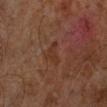Q: Was a biopsy performed?
A: imaged on a skin check; not biopsied
Q: How was this image acquired?
A: ~15 mm tile from a whole-body skin photo
Q: Where on the body is the lesion?
A: the left lower leg
Q: Who is the patient?
A: male, about 60 years old